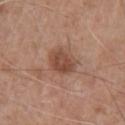The lesion was photographed on a routine skin check and not biopsied; there is no pathology result. A lesion tile, about 15 mm wide, cut from a 3D total-body photograph. A male subject, aged around 60. The lesion is on the chest. The recorded lesion diameter is about 3.5 mm. Imaged with white-light lighting.